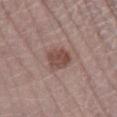{"biopsy_status": "not biopsied; imaged during a skin examination", "site": "right lower leg", "patient": {"sex": "female", "age_approx": 50}, "image": {"source": "total-body photography crop", "field_of_view_mm": 15}}